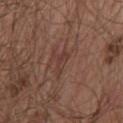  biopsy_status: not biopsied; imaged during a skin examination
  patient:
    sex: male
    age_approx: 65
  site: front of the torso
  image:
    source: total-body photography crop
    field_of_view_mm: 15
  lighting: white-light